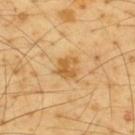No biopsy was performed on this lesion — it was imaged during a full skin examination and was not determined to be concerning. The total-body-photography lesion software estimated an area of roughly 5 mm², an outline eccentricity of about 0.45 (0 = round, 1 = elongated), and a shape-asymmetry score of about 0.3 (0 = symmetric). And it measured an average lesion color of about L≈60 a*≈20 b*≈47 (CIELAB) and about 10 CIELAB-L* units darker than the surrounding skin. The analysis additionally found border irregularity of about 3.5 on a 0–10 scale, internal color variation of about 2 on a 0–10 scale, and radial color variation of about 1. The software also gave a classifier nevus-likeness of about 40/100 and lesion-presence confidence of about 100/100. From the upper back. This image is a 15 mm lesion crop taken from a total-body photograph. The subject is a male aged 63 to 67. Measured at roughly 2.5 mm in maximum diameter.